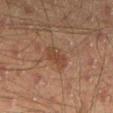biopsy status: imaged on a skin check; not biopsied
imaging modality: total-body-photography crop, ~15 mm field of view
body site: the leg
image-analysis metrics: a lesion-to-skin contrast of about 6 (normalized; higher = more distinct)
lighting: cross-polarized illumination
subject: male, aged approximately 45
diameter: ~3.5 mm (longest diameter)